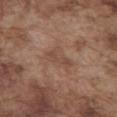notes: imaged on a skin check; not biopsied
patient: male, aged around 75
acquisition: ~15 mm tile from a whole-body skin photo
lesion size: about 4 mm
image-analysis metrics: a footprint of about 6.5 mm² and an eccentricity of roughly 0.8; a border-irregularity index near 4/10, a within-lesion color-variation index near 2.5/10, and a peripheral color-asymmetry measure near 1; a classifier nevus-likeness of about 0/100 and lesion-presence confidence of about 100/100
body site: the abdomen